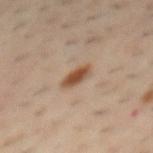Case summary:
– notes · total-body-photography surveillance lesion; no biopsy
– lighting · cross-polarized
– body site · the mid back
– subject · male, aged around 40
– acquisition · ~15 mm tile from a whole-body skin photo